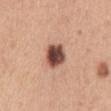A 15 mm crop from a total-body photograph taken for skin-cancer surveillance.
The lesion is located on the chest.
The subject is a female in their 30s.